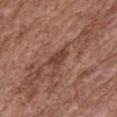A male patient in their mid- to late 70s.
The lesion is located on the mid back.
Captured under white-light illumination.
A 15 mm close-up extracted from a 3D total-body photography capture.